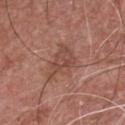biopsy status: no biopsy performed (imaged during a skin exam) | patient: male, roughly 55 years of age | TBP lesion metrics: a footprint of about 9.5 mm² and a shape-asymmetry score of about 0.4 (0 = symmetric); lesion-presence confidence of about 100/100 | image: ~15 mm tile from a whole-body skin photo | size: about 5 mm | anatomic site: the chest.